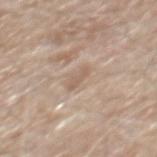Impression: Captured during whole-body skin photography for melanoma surveillance; the lesion was not biopsied. Context: Longest diameter approximately 2.5 mm. Located on the mid back. This image is a 15 mm lesion crop taken from a total-body photograph. A male subject, about 80 years old. The lesion-visualizer software estimated an area of roughly 2.5 mm². It also reported an average lesion color of about L≈59 a*≈15 b*≈27 (CIELAB), roughly 8 lightness units darker than nearby skin, and a lesion-to-skin contrast of about 5.5 (normalized; higher = more distinct). The software also gave a border-irregularity index near 4/10, a color-variation rating of about 0/10, and a peripheral color-asymmetry measure near 0. It also reported lesion-presence confidence of about 85/100. The tile uses white-light illumination.